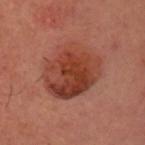Imaged during a routine full-body skin examination; the lesion was not biopsied and no histopathology is available.
This image is a 15 mm lesion crop taken from a total-body photograph.
The lesion is located on the chest.
A female subject, aged around 50.
Captured under cross-polarized illumination.